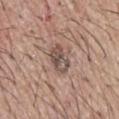follow-up: total-body-photography surveillance lesion; no biopsy | automated metrics: an average lesion color of about L≈50 a*≈16 b*≈22 (CIELAB), roughly 11 lightness units darker than nearby skin, and a normalized lesion–skin contrast near 8; a border-irregularity rating of about 2.5/10, a color-variation rating of about 6/10, and a peripheral color-asymmetry measure near 2; a lesion-detection confidence of about 100/100 | subject: male, aged 63–67 | imaging modality: ~15 mm crop, total-body skin-cancer survey | lesion diameter: ≈3.5 mm | illumination: white-light illumination | anatomic site: the back.